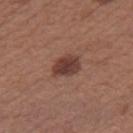Clinical impression:
Recorded during total-body skin imaging; not selected for excision or biopsy.
Clinical summary:
An algorithmic analysis of the crop reported an average lesion color of about L≈39 a*≈21 b*≈24 (CIELAB), roughly 12 lightness units darker than nearby skin, and a normalized lesion–skin contrast near 9.5. It also reported a within-lesion color-variation index near 3/10 and peripheral color asymmetry of about 1. The recorded lesion diameter is about 3.5 mm. This is a white-light tile. A 15 mm crop from a total-body photograph taken for skin-cancer surveillance. A female patient, in their 40s. The lesion is on the right thigh.